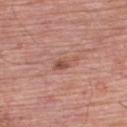Imaged with white-light lighting. A close-up tile cropped from a whole-body skin photograph, about 15 mm across. The recorded lesion diameter is about 3 mm. The lesion is located on the back. A male subject aged around 60.